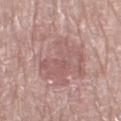- workup: total-body-photography surveillance lesion; no biopsy
- image: ~15 mm crop, total-body skin-cancer survey
- automated metrics: a lesion area of about 34 mm², a shape eccentricity near 0.75, and a shape-asymmetry score of about 0.25 (0 = symmetric); a mean CIELAB color near L≈58 a*≈20 b*≈21 and about 7 CIELAB-L* units darker than the surrounding skin; border irregularity of about 4 on a 0–10 scale, internal color variation of about 5 on a 0–10 scale, and peripheral color asymmetry of about 2; a nevus-likeness score of about 0/100
- diameter: about 8.5 mm
- location: the leg
- patient: female, roughly 70 years of age